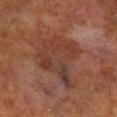The lesion was photographed on a routine skin check and not biopsied; there is no pathology result. Approximately 7 mm at its widest. A lesion tile, about 15 mm wide, cut from a 3D total-body photograph. The patient is a male aged around 70. The lesion is located on the right lower leg. The lesion-visualizer software estimated an area of roughly 22 mm², an eccentricity of roughly 0.65, and a shape-asymmetry score of about 0.4 (0 = symmetric). The software also gave about 7 CIELAB-L* units darker than the surrounding skin and a normalized lesion–skin contrast near 6. The analysis additionally found a border-irregularity rating of about 5/10 and a peripheral color-asymmetry measure near 2.5.